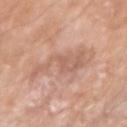Clinical summary:
This is a white-light tile. This image is a 15 mm lesion crop taken from a total-body photograph. The lesion's longest dimension is about 6.5 mm. From the right upper arm. A male patient roughly 80 years of age.